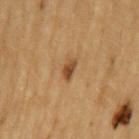{
  "biopsy_status": "not biopsied; imaged during a skin examination",
  "lighting": "cross-polarized",
  "image": {
    "source": "total-body photography crop",
    "field_of_view_mm": 15
  },
  "site": "left upper arm",
  "patient": {
    "sex": "male",
    "age_approx": "85+"
  },
  "lesion_size": {
    "long_diameter_mm_approx": 2.5
  }
}